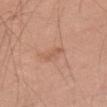The lesion is located on the right thigh.
The subject is a male in their mid- to late 60s.
A lesion tile, about 15 mm wide, cut from a 3D total-body photograph.